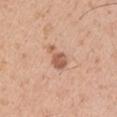Findings:
* notes — imaged on a skin check; not biopsied
* tile lighting — white-light illumination
* body site — the right upper arm
* imaging modality — 15 mm crop, total-body photography
* patient — male, aged approximately 30
* TBP lesion metrics — an outline eccentricity of about 0.8 (0 = round, 1 = elongated) and two-axis asymmetry of about 0.45; a lesion color around L≈59 a*≈23 b*≈31 in CIELAB, a lesion–skin lightness drop of about 12, and a normalized lesion–skin contrast near 7.5; a detector confidence of about 100 out of 100 that the crop contains a lesion
* lesion size — ~3.5 mm (longest diameter)